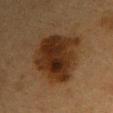Case summary:
• follow-up · no biopsy performed (imaged during a skin exam)
• automated lesion analysis · a lesion area of about 28 mm², an outline eccentricity of about 0.55 (0 = round, 1 = elongated), and two-axis asymmetry of about 0.25; a mean CIELAB color near L≈25 a*≈16 b*≈27, a lesion–skin lightness drop of about 11, and a lesion-to-skin contrast of about 12 (normalized; higher = more distinct); border irregularity of about 3 on a 0–10 scale, a within-lesion color-variation index near 6/10, and a peripheral color-asymmetry measure near 2; a classifier nevus-likeness of about 100/100
• lighting · cross-polarized illumination
• site · the right upper arm
• imaging modality · 15 mm crop, total-body photography
• patient · female, roughly 40 years of age